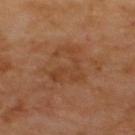Captured during whole-body skin photography for melanoma surveillance; the lesion was not biopsied.
A close-up tile cropped from a whole-body skin photograph, about 15 mm across.
A patient aged 63–67.
Located on the upper back.